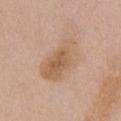Captured during whole-body skin photography for melanoma surveillance; the lesion was not biopsied.